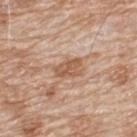Impression: The lesion was photographed on a routine skin check and not biopsied; there is no pathology result. Acquisition and patient details: The total-body-photography lesion software estimated a footprint of about 5.5 mm², an eccentricity of roughly 0.8, and a symmetry-axis asymmetry near 0.25. The software also gave an average lesion color of about L≈57 a*≈20 b*≈33 (CIELAB), about 10 CIELAB-L* units darker than the surrounding skin, and a normalized border contrast of about 7.5. The analysis additionally found lesion-presence confidence of about 100/100. Approximately 3.5 mm at its widest. A 15 mm close-up extracted from a 3D total-body photography capture. From the upper back. A male patient aged 78 to 82.